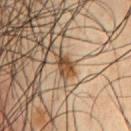This lesion was catalogued during total-body skin photography and was not selected for biopsy. This is a cross-polarized tile. A 15 mm close-up tile from a total-body photography series done for melanoma screening. A male patient, aged 58–62. Located on the chest. Automated tile analysis of the lesion measured an outline eccentricity of about 0.7 (0 = round, 1 = elongated) and two-axis asymmetry of about 0.35. The analysis additionally found an average lesion color of about L≈45 a*≈18 b*≈32 (CIELAB), about 15 CIELAB-L* units darker than the surrounding skin, and a normalized lesion–skin contrast near 11. The analysis additionally found a border-irregularity index near 3.5/10, a within-lesion color-variation index near 3/10, and a peripheral color-asymmetry measure near 1. The analysis additionally found an automated nevus-likeness rating near 80 out of 100.